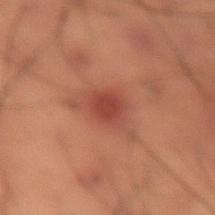Findings:
* biopsy status: total-body-photography surveillance lesion; no biopsy
* acquisition: ~15 mm crop, total-body skin-cancer survey
* subject: male, aged around 50
* automated lesion analysis: a footprint of about 5.5 mm²; a border-irregularity rating of about 1.5/10, internal color variation of about 1.5 on a 0–10 scale, and a peripheral color-asymmetry measure near 0.5; a classifier nevus-likeness of about 80/100 and a lesion-detection confidence of about 100/100
* lighting: cross-polarized
* location: the lower back